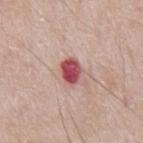Impression:
This lesion was catalogued during total-body skin photography and was not selected for biopsy.
Acquisition and patient details:
Measured at roughly 3 mm in maximum diameter. A male subject, about 65 years old. Located on the abdomen. A lesion tile, about 15 mm wide, cut from a 3D total-body photograph.